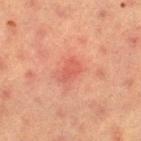{
  "biopsy_status": "not biopsied; imaged during a skin examination",
  "lighting": "cross-polarized",
  "site": "left thigh",
  "image": {
    "source": "total-body photography crop",
    "field_of_view_mm": 15
  },
  "patient": {
    "sex": "female",
    "age_approx": 55
  }
}